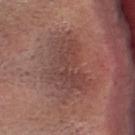Context: Captured under white-light illumination. A roughly 15 mm field-of-view crop from a total-body skin photograph. Measured at roughly 7.5 mm in maximum diameter. Automated tile analysis of the lesion measured a border-irregularity index near 5/10 and internal color variation of about 4.5 on a 0–10 scale. The lesion is located on the head or neck. The patient is a female aged approximately 25.